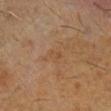Recorded during total-body skin imaging; not selected for excision or biopsy.
Measured at roughly 2 mm in maximum diameter.
The total-body-photography lesion software estimated a footprint of about 2 mm² and an outline eccentricity of about 0.8 (0 = round, 1 = elongated). The software also gave roughly 4 lightness units darker than nearby skin and a normalized lesion–skin contrast near 4.
A region of skin cropped from a whole-body photographic capture, roughly 15 mm wide.
Imaged with cross-polarized lighting.
On the right lower leg.
A male patient in their 60s.